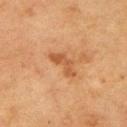Case summary:
– biopsy status: imaged on a skin check; not biopsied
– patient: female, aged around 55
– tile lighting: cross-polarized
– size: about 3.5 mm
– location: the left upper arm
– acquisition: ~15 mm crop, total-body skin-cancer survey